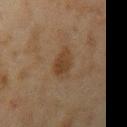{
  "biopsy_status": "not biopsied; imaged during a skin examination",
  "image": {
    "source": "total-body photography crop",
    "field_of_view_mm": 15
  },
  "site": "right upper arm",
  "patient": {
    "sex": "male",
    "age_approx": 45
  }
}